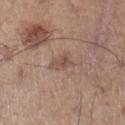Q: Is there a histopathology result?
A: no biopsy performed (imaged during a skin exam)
Q: What is the imaging modality?
A: total-body-photography crop, ~15 mm field of view
Q: What is the anatomic site?
A: the left lower leg
Q: Patient demographics?
A: male, about 60 years old
Q: How large is the lesion?
A: about 3 mm
Q: What lighting was used for the tile?
A: white-light